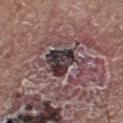Recorded during total-body skin imaging; not selected for excision or biopsy. The lesion is on the right lower leg. The tile uses white-light illumination. Longest diameter approximately 6 mm. Automated tile analysis of the lesion measured a lesion area of about 11 mm², an outline eccentricity of about 0.8 (0 = round, 1 = elongated), and a shape-asymmetry score of about 0.4 (0 = symmetric). And it measured a lesion-detection confidence of about 70/100. The patient is a male in their mid-60s. A 15 mm close-up extracted from a 3D total-body photography capture.